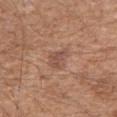Impression: This lesion was catalogued during total-body skin photography and was not selected for biopsy. Clinical summary: The tile uses white-light illumination. Automated image analysis of the tile measured a nevus-likeness score of about 0/100 and lesion-presence confidence of about 100/100. The lesion is on the right upper arm. A male patient roughly 60 years of age. A close-up tile cropped from a whole-body skin photograph, about 15 mm across. About 4 mm across.